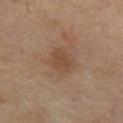{"image": {"source": "total-body photography crop", "field_of_view_mm": 15}, "patient": {"sex": "female", "age_approx": 50}, "automated_metrics": {"area_mm2_approx": 6.0, "eccentricity": 0.65, "shape_asymmetry": 0.2, "cielab_L": 44, "cielab_a": 17, "cielab_b": 30, "vs_skin_darker_L": 6.0, "vs_skin_contrast_norm": 6.0, "nevus_likeness_0_100": 0, "lesion_detection_confidence_0_100": 100}, "site": "abdomen", "lesion_size": {"long_diameter_mm_approx": 3.5}, "lighting": "cross-polarized"}